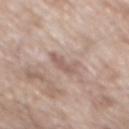* notes — no biopsy performed (imaged during a skin exam)